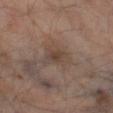| field | value |
|---|---|
| notes | imaged on a skin check; not biopsied |
| image source | 15 mm crop, total-body photography |
| size | ~2.5 mm (longest diameter) |
| patient | male, roughly 65 years of age |
| illumination | cross-polarized |
| anatomic site | the left thigh |